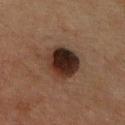Recorded during total-body skin imaging; not selected for excision or biopsy. The subject is a male roughly 65 years of age. A region of skin cropped from a whole-body photographic capture, roughly 15 mm wide. An algorithmic analysis of the crop reported an area of roughly 13 mm², an eccentricity of roughly 0.3, and a symmetry-axis asymmetry near 0.1. And it measured a lesion–skin lightness drop of about 14. And it measured border irregularity of about 1.5 on a 0–10 scale, internal color variation of about 7.5 on a 0–10 scale, and peripheral color asymmetry of about 2. The software also gave a nevus-likeness score of about 80/100 and a lesion-detection confidence of about 100/100. The lesion is located on the upper back.